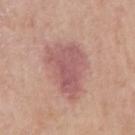Assessment:
Recorded during total-body skin imaging; not selected for excision or biopsy.
Acquisition and patient details:
This is a white-light tile. The patient is a male aged around 75. The recorded lesion diameter is about 6.5 mm. An algorithmic analysis of the crop reported a footprint of about 20 mm² and an eccentricity of roughly 0.6. The analysis additionally found an average lesion color of about L≈56 a*≈24 b*≈22 (CIELAB) and a normalized border contrast of about 7. The software also gave a border-irregularity rating of about 4/10, internal color variation of about 3.5 on a 0–10 scale, and a peripheral color-asymmetry measure near 1. The analysis additionally found a classifier nevus-likeness of about 20/100 and lesion-presence confidence of about 100/100. A 15 mm crop from a total-body photograph taken for skin-cancer surveillance. The lesion is located on the right upper arm.